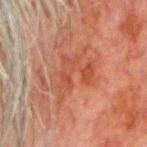Assessment:
No biopsy was performed on this lesion — it was imaged during a full skin examination and was not determined to be concerning.
Image and clinical context:
A male patient, aged 78–82. A lesion tile, about 15 mm wide, cut from a 3D total-body photograph. The lesion is on the head or neck. Imaged with cross-polarized lighting. The recorded lesion diameter is about 5.5 mm.